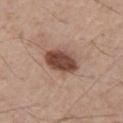The lesion was tiled from a total-body skin photograph and was not biopsied. A male patient in their mid- to late 50s. The recorded lesion diameter is about 4 mm. Imaged with white-light lighting. Located on the chest. This image is a 15 mm lesion crop taken from a total-body photograph.